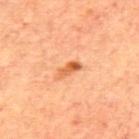Impression: The lesion was photographed on a routine skin check and not biopsied; there is no pathology result. Image and clinical context: Located on the back. The recorded lesion diameter is about 3 mm. A lesion tile, about 15 mm wide, cut from a 3D total-body photograph. The tile uses cross-polarized illumination. The subject is a male aged 68 to 72.